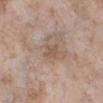Assessment: The lesion was photographed on a routine skin check and not biopsied; there is no pathology result. Image and clinical context: A female subject, about 55 years old. The lesion is on the right lower leg. A 15 mm close-up extracted from a 3D total-body photography capture. The lesion-visualizer software estimated an area of roughly 3.5 mm² and two-axis asymmetry of about 0.25. The analysis additionally found a mean CIELAB color near L≈54 a*≈16 b*≈27, a lesion–skin lightness drop of about 7, and a normalized lesion–skin contrast near 5.5.